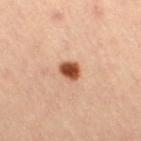Case summary:
- notes: imaged on a skin check; not biopsied
- tile lighting: cross-polarized illumination
- lesion diameter: about 2.5 mm
- image source: ~15 mm crop, total-body skin-cancer survey
- subject: female, in their mid- to late 40s
- site: the right thigh